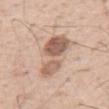Findings:
– biopsy status: total-body-photography surveillance lesion; no biopsy
– diameter: about 7 mm
– image source: 15 mm crop, total-body photography
– location: the abdomen
– patient: male, roughly 55 years of age
– TBP lesion metrics: a border-irregularity rating of about 5.5/10, a within-lesion color-variation index near 5.5/10, and a peripheral color-asymmetry measure near 1.5
– tile lighting: white-light illumination